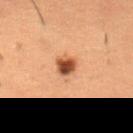Q: How was this image acquired?
A: ~15 mm tile from a whole-body skin photo
Q: Patient demographics?
A: male, aged 48 to 52
Q: What did automated image analysis measure?
A: a color-variation rating of about 5/10 and radial color variation of about 1.5; a nevus-likeness score of about 100/100 and lesion-presence confidence of about 100/100
Q: What is the anatomic site?
A: the abdomen
Q: What lighting was used for the tile?
A: cross-polarized